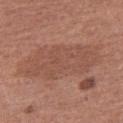Impression: Recorded during total-body skin imaging; not selected for excision or biopsy. Clinical summary: Cropped from a total-body skin-imaging series; the visible field is about 15 mm. A female subject roughly 50 years of age. Captured under white-light illumination. From the right thigh.